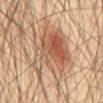<lesion>
<biopsy_status>not biopsied; imaged during a skin examination</biopsy_status>
<patient>
  <sex>male</sex>
  <age_approx>60</age_approx>
</patient>
<image>
  <source>total-body photography crop</source>
  <field_of_view_mm>15</field_of_view_mm>
</image>
<site>abdomen</site>
<lighting>cross-polarized</lighting>
<lesion_size>
  <long_diameter_mm_approx>8.5</long_diameter_mm_approx>
</lesion_size>
</lesion>